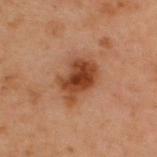biopsy_status: not biopsied; imaged during a skin examination
image:
  source: total-body photography crop
  field_of_view_mm: 15
lighting: cross-polarized
lesion_size:
  long_diameter_mm_approx: 4.5
site: back
patient:
  sex: male
  age_approx: 55